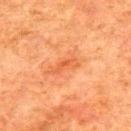patient: male, aged approximately 80; location: the upper back; acquisition: ~15 mm tile from a whole-body skin photo.